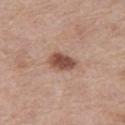  biopsy_status: not biopsied; imaged during a skin examination
  image:
    source: total-body photography crop
    field_of_view_mm: 15
  automated_metrics:
    eccentricity: 0.8
    shape_asymmetry: 0.25
    cielab_L: 50
    cielab_a: 21
    cielab_b: 26
    vs_skin_contrast_norm: 10.0
  patient:
    sex: female
    age_approx: 40
  lighting: white-light
  site: left thigh